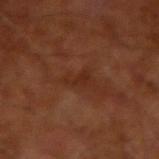Captured during whole-body skin photography for melanoma surveillance; the lesion was not biopsied.
A 15 mm close-up tile from a total-body photography series done for melanoma screening.
A male subject aged 63 to 67.
Captured under cross-polarized illumination.
From the right upper arm.
Approximately 2.5 mm at its widest.